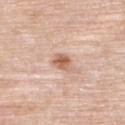biopsy status: imaged on a skin check; not biopsied | patient: female, aged 63–67 | automated lesion analysis: a color-variation rating of about 4/10 and peripheral color asymmetry of about 1.5; a classifier nevus-likeness of about 90/100 and a lesion-detection confidence of about 100/100 | location: the upper back | size: ≈2.5 mm | image source: 15 mm crop, total-body photography | illumination: white-light illumination.